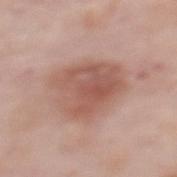notes: total-body-photography surveillance lesion; no biopsy | location: the upper back | tile lighting: white-light illumination | patient: female, aged around 50 | image source: 15 mm crop, total-body photography.